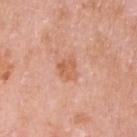Recorded during total-body skin imaging; not selected for excision or biopsy.
The patient is a male about 60 years old.
The lesion is located on the arm.
A 15 mm close-up tile from a total-body photography series done for melanoma screening.
Imaged with white-light lighting.
Approximately 2.5 mm at its widest.